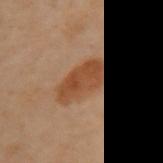Impression:
Imaged during a routine full-body skin examination; the lesion was not biopsied and no histopathology is available.
Clinical summary:
On the arm. The tile uses cross-polarized illumination. Measured at roughly 5.5 mm in maximum diameter. A female patient in their 60s. Cropped from a total-body skin-imaging series; the visible field is about 15 mm.